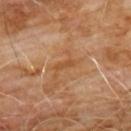{"biopsy_status": "not biopsied; imaged during a skin examination", "automated_metrics": {"area_mm2_approx": 2.0, "cielab_L": 48, "cielab_a": 22, "cielab_b": 37, "vs_skin_darker_L": 7.0, "vs_skin_contrast_norm": 6.0, "color_variation_0_10": 0.0}, "lesion_size": {"long_diameter_mm_approx": 2.5}, "lighting": "cross-polarized", "site": "chest", "image": {"source": "total-body photography crop", "field_of_view_mm": 15}, "patient": {"sex": "male", "age_approx": 60}}